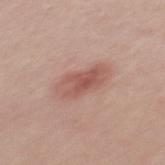Clinical impression:
This lesion was catalogued during total-body skin photography and was not selected for biopsy.
Clinical summary:
An algorithmic analysis of the crop reported a lesion color around L≈55 a*≈23 b*≈25 in CIELAB, roughly 10 lightness units darker than nearby skin, and a normalized border contrast of about 7. The software also gave border irregularity of about 2.5 on a 0–10 scale, a color-variation rating of about 4.5/10, and peripheral color asymmetry of about 1.5. The patient is a male in their mid-20s. Located on the upper back. A close-up tile cropped from a whole-body skin photograph, about 15 mm across.